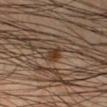Image and clinical context: On the right lower leg. A lesion tile, about 15 mm wide, cut from a 3D total-body photograph. An algorithmic analysis of the crop reported a lesion area of about 3 mm², an outline eccentricity of about 0.85 (0 = round, 1 = elongated), and a shape-asymmetry score of about 0.3 (0 = symmetric). The software also gave about 9 CIELAB-L* units darker than the surrounding skin and a normalized lesion–skin contrast near 9. A male patient approximately 50 years of age. Longest diameter approximately 2.5 mm.